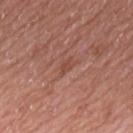{
  "biopsy_status": "not biopsied; imaged during a skin examination",
  "site": "upper back",
  "lighting": "white-light",
  "lesion_size": {
    "long_diameter_mm_approx": 2.5
  },
  "image": {
    "source": "total-body photography crop",
    "field_of_view_mm": 15
  },
  "patient": {
    "sex": "male",
    "age_approx": 65
  }
}